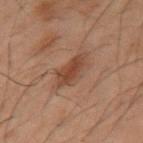Imaged during a routine full-body skin examination; the lesion was not biopsied and no histopathology is available. The lesion is located on the mid back. Imaged with cross-polarized lighting. A 15 mm crop from a total-body photograph taken for skin-cancer surveillance. The subject is a male about 50 years old. Approximately 4 mm at its widest. An algorithmic analysis of the crop reported a footprint of about 7.5 mm², an outline eccentricity of about 0.75 (0 = round, 1 = elongated), and a symmetry-axis asymmetry near 0.25.